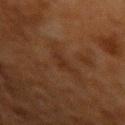Clinical impression: The lesion was tiled from a total-body skin photograph and was not biopsied. Image and clinical context: Cropped from a whole-body photographic skin survey; the tile spans about 15 mm. About 2.5 mm across. Automated tile analysis of the lesion measured an area of roughly 3 mm², an outline eccentricity of about 0.8 (0 = round, 1 = elongated), and a shape-asymmetry score of about 0.4 (0 = symmetric). The lesion is located on the left forearm. A male subject in their 60s.